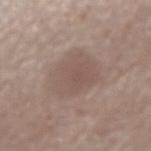{"biopsy_status": "not biopsied; imaged during a skin examination", "site": "right forearm", "image": {"source": "total-body photography crop", "field_of_view_mm": 15}, "patient": {"sex": "female", "age_approx": 65}, "lighting": "white-light"}